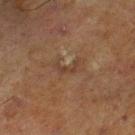Case summary:
- biopsy status — total-body-photography surveillance lesion; no biopsy
- body site — the right lower leg
- image source — total-body-photography crop, ~15 mm field of view
- tile lighting — cross-polarized illumination
- diameter — ≈3 mm
- patient — male, roughly 70 years of age
- TBP lesion metrics — border irregularity of about 8 on a 0–10 scale and radial color variation of about 0; an automated nevus-likeness rating near 0 out of 100